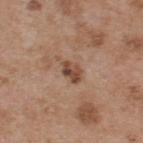Clinical summary:
About 3 mm across. The total-body-photography lesion software estimated an eccentricity of roughly 0.75 and a shape-asymmetry score of about 0.2 (0 = symmetric). The software also gave an average lesion color of about L≈47 a*≈20 b*≈28 (CIELAB), about 11 CIELAB-L* units darker than the surrounding skin, and a lesion-to-skin contrast of about 8.5 (normalized; higher = more distinct). The analysis additionally found a within-lesion color-variation index near 5/10. The software also gave a classifier nevus-likeness of about 30/100 and a lesion-detection confidence of about 100/100. A 15 mm close-up extracted from a 3D total-body photography capture. On the upper back. A male subject, aged 53 to 57. This is a white-light tile.